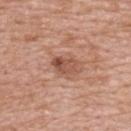workup — no biopsy performed (imaged during a skin exam); imaging modality — 15 mm crop, total-body photography; body site — the upper back; subject — female, in their 60s.